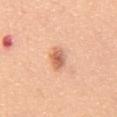notes: imaged on a skin check; not biopsied
location: the abdomen
patient: male, approximately 50 years of age
illumination: white-light
imaging modality: ~15 mm tile from a whole-body skin photo